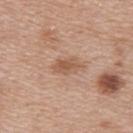Case summary:
- image source — ~15 mm tile from a whole-body skin photo
- lighting — white-light illumination
- subject — female, approximately 40 years of age
- anatomic site — the upper back
- image-analysis metrics — a footprint of about 5 mm² and a symmetry-axis asymmetry near 0.3; a mean CIELAB color near L≈56 a*≈20 b*≈31, about 9 CIELAB-L* units darker than the surrounding skin, and a normalized border contrast of about 6.5; internal color variation of about 2 on a 0–10 scale; a classifier nevus-likeness of about 0/100 and a lesion-detection confidence of about 100/100
- size — about 3.5 mm